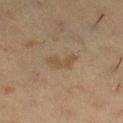follow-up=catalogued during a skin exam; not biopsied
anatomic site=the right lower leg
subject=female, aged around 40
lesion size=about 3.5 mm
imaging modality=~15 mm tile from a whole-body skin photo